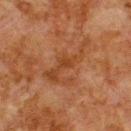<case>
  <biopsy_status>not biopsied; imaged during a skin examination</biopsy_status>
  <lesion_size>
    <long_diameter_mm_approx>7.0</long_diameter_mm_approx>
  </lesion_size>
  <image>
    <source>total-body photography crop</source>
    <field_of_view_mm>15</field_of_view_mm>
  </image>
  <lighting>cross-polarized</lighting>
  <patient>
    <sex>male</sex>
    <age_approx>80</age_approx>
  </patient>
  <site>upper back</site>
  <automated_metrics>
    <area_mm2_approx>11.0</area_mm2_approx>
    <eccentricity>0.9</eccentricity>
    <shape_asymmetry>0.6</shape_asymmetry>
    <vs_skin_darker_L>6.0</vs_skin_darker_L>
    <vs_skin_contrast_norm>6.0</vs_skin_contrast_norm>
    <border_irregularity_0_10>9.5</border_irregularity_0_10>
    <color_variation_0_10>1.5</color_variation_0_10>
  </automated_metrics>
</case>